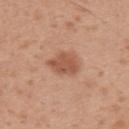Assessment:
Part of a total-body skin-imaging series; this lesion was reviewed on a skin check and was not flagged for biopsy.